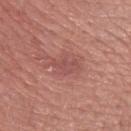Case summary:
* workup: imaged on a skin check; not biopsied
* size: about 4 mm
* tile lighting: white-light illumination
* site: the right forearm
* image source: ~15 mm tile from a whole-body skin photo
* patient: female, aged around 50